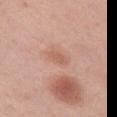This lesion was catalogued during total-body skin photography and was not selected for biopsy.
From the upper back.
A roughly 15 mm field-of-view crop from a total-body skin photograph.
A female patient, aged 48 to 52.
The tile uses white-light illumination.
An algorithmic analysis of the crop reported an average lesion color of about L≈59 a*≈23 b*≈30 (CIELAB) and about 7 CIELAB-L* units darker than the surrounding skin. The analysis additionally found a border-irregularity index near 3/10, a color-variation rating of about 1/10, and radial color variation of about 0.